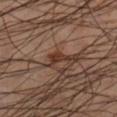Q: Was this lesion biopsied?
A: total-body-photography surveillance lesion; no biopsy
Q: What are the patient's age and sex?
A: male, aged around 50
Q: Lesion location?
A: the right lower leg
Q: Lesion size?
A: about 2.5 mm
Q: What kind of image is this?
A: ~15 mm crop, total-body skin-cancer survey
Q: What lighting was used for the tile?
A: cross-polarized